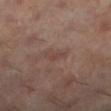Q: Was a biopsy performed?
A: imaged on a skin check; not biopsied
Q: Patient demographics?
A: female, aged approximately 60
Q: What did automated image analysis measure?
A: a lesion area of about 3 mm²; a border-irregularity rating of about 4/10, a within-lesion color-variation index near 0.5/10, and a peripheral color-asymmetry measure near 0.5
Q: What kind of image is this?
A: ~15 mm tile from a whole-body skin photo
Q: What is the anatomic site?
A: the left lower leg
Q: How was the tile lit?
A: cross-polarized
Q: What is the lesion's diameter?
A: ~3 mm (longest diameter)